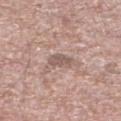<case>
<biopsy_status>not biopsied; imaged during a skin examination</biopsy_status>
<image>
  <source>total-body photography crop</source>
  <field_of_view_mm>15</field_of_view_mm>
</image>
<site>left lower leg</site>
<patient>
  <sex>male</sex>
  <age_approx>60</age_approx>
</patient>
</case>